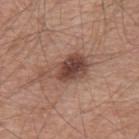{"biopsy_status": "not biopsied; imaged during a skin examination", "site": "back", "automated_metrics": {"area_mm2_approx": 11.0, "shape_asymmetry": 0.35, "border_irregularity_0_10": 4.0, "color_variation_0_10": 7.0, "peripheral_color_asymmetry": 2.5, "nevus_likeness_0_100": 75, "lesion_detection_confidence_0_100": 100}, "image": {"source": "total-body photography crop", "field_of_view_mm": 15}, "patient": {"sex": "male", "age_approx": 55}, "lighting": "white-light", "lesion_size": {"long_diameter_mm_approx": 5.5}}